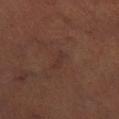<record>
<biopsy_status>not biopsied; imaged during a skin examination</biopsy_status>
<lighting>cross-polarized</lighting>
<automated_metrics>
  <eccentricity>0.9</eccentricity>
  <shape_asymmetry>0.35</shape_asymmetry>
  <cielab_L>31</cielab_L>
  <cielab_a>19</cielab_a>
  <cielab_b>21</cielab_b>
  <border_irregularity_0_10>4.0</border_irregularity_0_10>
  <color_variation_0_10>0.0</color_variation_0_10>
  <peripheral_color_asymmetry>0.0</peripheral_color_asymmetry>
</automated_metrics>
<image>
  <source>total-body photography crop</source>
  <field_of_view_mm>15</field_of_view_mm>
</image>
<patient>
  <sex>male</sex>
  <age_approx>50</age_approx>
</patient>
<lesion_size>
  <long_diameter_mm_approx>2.5</long_diameter_mm_approx>
</lesion_size>
<site>leg</site>
</record>